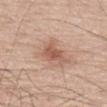{"biopsy_status": "not biopsied; imaged during a skin examination", "automated_metrics": {"area_mm2_approx": 6.5, "shape_asymmetry": 0.35, "border_irregularity_0_10": 3.5, "color_variation_0_10": 3.0, "peripheral_color_asymmetry": 1.0, "nevus_likeness_0_100": 30, "lesion_detection_confidence_0_100": 100}, "lighting": "white-light", "image": {"source": "total-body photography crop", "field_of_view_mm": 15}, "lesion_size": {"long_diameter_mm_approx": 4.0}, "site": "upper back", "patient": {"sex": "male", "age_approx": 70}}